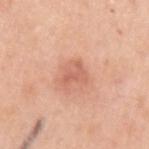Q: What are the patient's age and sex?
A: male, approximately 50 years of age
Q: What is the lesion's diameter?
A: ~3 mm (longest diameter)
Q: How was the tile lit?
A: white-light illumination
Q: Automated lesion metrics?
A: an area of roughly 4 mm² and a shape eccentricity near 0.8; a lesion color around L≈62 a*≈27 b*≈32 in CIELAB, about 9 CIELAB-L* units darker than the surrounding skin, and a normalized lesion–skin contrast near 5.5; a classifier nevus-likeness of about 25/100 and lesion-presence confidence of about 100/100
Q: Lesion location?
A: the left upper arm
Q: How was this image acquired?
A: total-body-photography crop, ~15 mm field of view